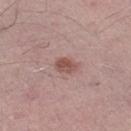The tile uses white-light illumination. From the left thigh. A male patient aged 58 to 62. Cropped from a total-body skin-imaging series; the visible field is about 15 mm.